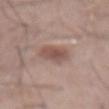Imaged during a routine full-body skin examination; the lesion was not biopsied and no histopathology is available.
Longest diameter approximately 3.5 mm.
A roughly 15 mm field-of-view crop from a total-body skin photograph.
On the front of the torso.
This is a white-light tile.
The lesion-visualizer software estimated a lesion color around L≈52 a*≈19 b*≈23 in CIELAB and about 10 CIELAB-L* units darker than the surrounding skin. The analysis additionally found a within-lesion color-variation index near 4/10. And it measured a classifier nevus-likeness of about 85/100.
A male subject, aged 68 to 72.